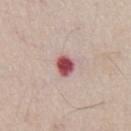No biopsy was performed on this lesion — it was imaged during a full skin examination and was not determined to be concerning. About 2.5 mm across. A close-up tile cropped from a whole-body skin photograph, about 15 mm across. A male subject, about 65 years old. From the front of the torso. The lesion-visualizer software estimated a lesion area of about 4.5 mm², a shape eccentricity near 0.6, and a symmetry-axis asymmetry near 0.2. The software also gave a nevus-likeness score of about 0/100. The tile uses white-light illumination.